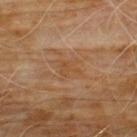follow-up: imaged on a skin check; not biopsied | automated metrics: a lesion-detection confidence of about 95/100 | illumination: cross-polarized illumination | body site: the chest | patient: male, roughly 60 years of age | acquisition: total-body-photography crop, ~15 mm field of view.